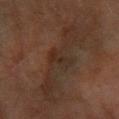| feature | finding |
|---|---|
| acquisition | ~15 mm crop, total-body skin-cancer survey |
| patient | female, aged approximately 70 |
| lesion diameter | ≈3.5 mm |
| anatomic site | the left forearm |
| tile lighting | cross-polarized illumination |